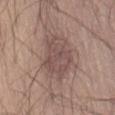{
  "lesion_size": {
    "long_diameter_mm_approx": 6.0
  },
  "image": {
    "source": "total-body photography crop",
    "field_of_view_mm": 15
  },
  "lighting": "white-light",
  "patient": {
    "sex": "male",
    "age_approx": 45
  },
  "site": "abdomen"
}